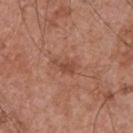The lesion was photographed on a routine skin check and not biopsied; there is no pathology result.
About 3 mm across.
The lesion is on the front of the torso.
The patient is a male aged around 55.
A close-up tile cropped from a whole-body skin photograph, about 15 mm across.